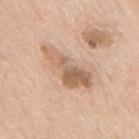notes — imaged on a skin check; not biopsied
patient — male, approximately 65 years of age
site — the mid back
image — ~15 mm tile from a whole-body skin photo
TBP lesion metrics — a mean CIELAB color near L≈62 a*≈18 b*≈31, about 11 CIELAB-L* units darker than the surrounding skin, and a lesion-to-skin contrast of about 7 (normalized; higher = more distinct); a classifier nevus-likeness of about 0/100 and a detector confidence of about 100 out of 100 that the crop contains a lesion
tile lighting — white-light
lesion size — about 7 mm